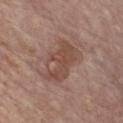{"biopsy_status": "not biopsied; imaged during a skin examination", "lighting": "white-light", "site": "chest", "lesion_size": {"long_diameter_mm_approx": 5.5}, "patient": {"sex": "male", "age_approx": 65}, "image": {"source": "total-body photography crop", "field_of_view_mm": 15}}